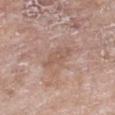<lesion>
<biopsy_status>not biopsied; imaged during a skin examination</biopsy_status>
<image>
  <source>total-body photography crop</source>
  <field_of_view_mm>15</field_of_view_mm>
</image>
<site>chest</site>
<patient>
  <sex>female</sex>
  <age_approx>75</age_approx>
</patient>
<lighting>white-light</lighting>
<lesion_size>
  <long_diameter_mm_approx>4.0</long_diameter_mm_approx>
</lesion_size>
<automated_metrics>
  <area_mm2_approx>6.5</area_mm2_approx>
  <eccentricity>0.85</eccentricity>
  <vs_skin_darker_L>7.0</vs_skin_darker_L>
  <vs_skin_contrast_norm>5.0</vs_skin_contrast_norm>
  <nevus_likeness_0_100>0</nevus_likeness_0_100>
</automated_metrics>
</lesion>